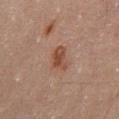Case summary:
* workup — catalogued during a skin exam; not biopsied
* subject — male, aged around 60
* lesion diameter — ~3.5 mm (longest diameter)
* imaging modality — 15 mm crop, total-body photography
* site — the abdomen
* tile lighting — cross-polarized illumination